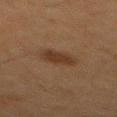The lesion was tiled from a total-body skin photograph and was not biopsied.
An algorithmic analysis of the crop reported a lesion area of about 5.5 mm², a shape eccentricity near 0.85, and a symmetry-axis asymmetry near 0.25.
The recorded lesion diameter is about 3.5 mm.
A male patient in their mid- to late 60s.
A close-up tile cropped from a whole-body skin photograph, about 15 mm across.
The lesion is located on the mid back.